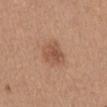– workup: imaged on a skin check; not biopsied
– image source: 15 mm crop, total-body photography
– anatomic site: the abdomen
– size: ≈3.5 mm
– patient: female, aged around 55
– illumination: white-light